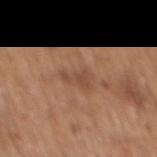| field | value |
|---|---|
| biopsy status | catalogued during a skin exam; not biopsied |